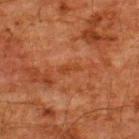biopsy_status: not biopsied; imaged during a skin examination
automated_metrics:
  eccentricity: 0.95
  shape_asymmetry: 0.35
  border_irregularity_0_10: 4.0
  color_variation_0_10: 0.0
  peripheral_color_asymmetry: 0.0
site: upper back
patient:
  sex: male
  age_approx: 60
lesion_size:
  long_diameter_mm_approx: 2.5
image:
  source: total-body photography crop
  field_of_view_mm: 15